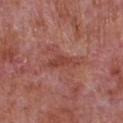| key | value |
|---|---|
| notes | no biopsy performed (imaged during a skin exam) |
| acquisition | total-body-photography crop, ~15 mm field of view |
| diameter | ≈4 mm |
| lighting | white-light illumination |
| location | the chest |
| patient | male, aged approximately 65 |